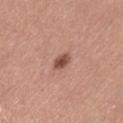Clinical impression:
No biopsy was performed on this lesion — it was imaged during a full skin examination and was not determined to be concerning.
Image and clinical context:
The lesion is located on the left thigh. A 15 mm crop from a total-body photograph taken for skin-cancer surveillance. A female subject about 35 years old.